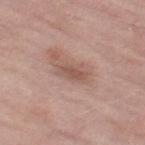• biopsy status: catalogued during a skin exam; not biopsied
• diameter: ~3.5 mm (longest diameter)
• imaging modality: ~15 mm tile from a whole-body skin photo
• anatomic site: the right thigh
• patient: female, aged approximately 70